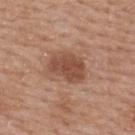Case summary:
- notes: no biopsy performed (imaged during a skin exam)
- location: the back
- patient: female, roughly 60 years of age
- automated metrics: about 11 CIELAB-L* units darker than the surrounding skin and a normalized lesion–skin contrast near 8; an automated nevus-likeness rating near 25 out of 100 and a detector confidence of about 100 out of 100 that the crop contains a lesion
- lesion size: ~4.5 mm (longest diameter)
- tile lighting: white-light illumination
- image source: ~15 mm crop, total-body skin-cancer survey